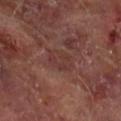Assessment:
Part of a total-body skin-imaging series; this lesion was reviewed on a skin check and was not flagged for biopsy.
Acquisition and patient details:
The patient is a male aged around 65. A 15 mm close-up tile from a total-body photography series done for melanoma screening. The recorded lesion diameter is about 3.5 mm. Located on the right forearm. Automated tile analysis of the lesion measured an eccentricity of roughly 0.7 and two-axis asymmetry of about 0.2. And it measured lesion-presence confidence of about 90/100.